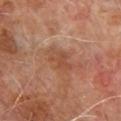This lesion was catalogued during total-body skin photography and was not selected for biopsy. Located on the chest. The total-body-photography lesion software estimated a footprint of about 7.5 mm², an outline eccentricity of about 0.8 (0 = round, 1 = elongated), and a shape-asymmetry score of about 0.25 (0 = symmetric). It also reported border irregularity of about 3.5 on a 0–10 scale and a within-lesion color-variation index near 3.5/10. A lesion tile, about 15 mm wide, cut from a 3D total-body photograph. About 4 mm across. A male patient, aged approximately 65. Imaged with cross-polarized lighting.